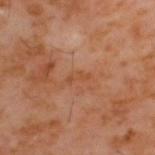biopsy status — no biopsy performed (imaged during a skin exam) | size — ~2.5 mm (longest diameter) | imaging modality — total-body-photography crop, ~15 mm field of view | subject — male, roughly 60 years of age | body site — the upper back.